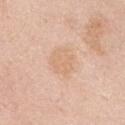<case>
<biopsy_status>not biopsied; imaged during a skin examination</biopsy_status>
<patient>
  <sex>female</sex>
  <age_approx>65</age_approx>
</patient>
<image>
  <source>total-body photography crop</source>
  <field_of_view_mm>15</field_of_view_mm>
</image>
<site>abdomen</site>
<lighting>white-light</lighting>
</case>